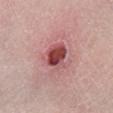follow-up: catalogued during a skin exam; not biopsied
site: the right lower leg
imaging modality: total-body-photography crop, ~15 mm field of view
automated lesion analysis: an average lesion color of about L≈47 a*≈31 b*≈22 (CIELAB); a nevus-likeness score of about 80/100 and a detector confidence of about 100 out of 100 that the crop contains a lesion
size: ~3.5 mm (longest diameter)
illumination: white-light
subject: male, aged around 60